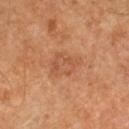The lesion was photographed on a routine skin check and not biopsied; there is no pathology result.
The lesion's longest dimension is about 4.5 mm.
A 15 mm close-up extracted from a 3D total-body photography capture.
Automated image analysis of the tile measured an eccentricity of roughly 0.8. It also reported an automated nevus-likeness rating near 0 out of 100 and a detector confidence of about 100 out of 100 that the crop contains a lesion.
The patient is a male about 60 years old.
The lesion is on the leg.